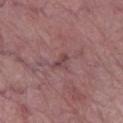Part of a total-body skin-imaging series; this lesion was reviewed on a skin check and was not flagged for biopsy.
A roughly 15 mm field-of-view crop from a total-body skin photograph.
A female subject about 65 years old.
About 2.5 mm across.
The tile uses white-light illumination.
The lesion is located on the left thigh.
Automated image analysis of the tile measured an automated nevus-likeness rating near 0 out of 100 and lesion-presence confidence of about 55/100.